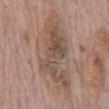{
  "biopsy_status": "not biopsied; imaged during a skin examination",
  "site": "abdomen",
  "lighting": "white-light",
  "image": {
    "source": "total-body photography crop",
    "field_of_view_mm": 15
  },
  "patient": {
    "sex": "male",
    "age_approx": 70
  },
  "lesion_size": {
    "long_diameter_mm_approx": 12.0
  }
}